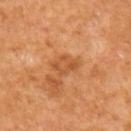follow-up: catalogued during a skin exam; not biopsied | TBP lesion metrics: a footprint of about 4.5 mm² and an outline eccentricity of about 0.8 (0 = round, 1 = elongated); border irregularity of about 3.5 on a 0–10 scale, a within-lesion color-variation index near 2.5/10, and peripheral color asymmetry of about 1; a detector confidence of about 100 out of 100 that the crop contains a lesion | location: the upper back | image: ~15 mm tile from a whole-body skin photo | diameter: about 3 mm | illumination: cross-polarized illumination | subject: female, aged around 55.